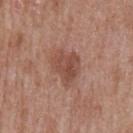Recorded during total-body skin imaging; not selected for excision or biopsy.
On the mid back.
Automated image analysis of the tile measured an eccentricity of roughly 0.65 and two-axis asymmetry of about 0.35.
Cropped from a whole-body photographic skin survey; the tile spans about 15 mm.
Approximately 4 mm at its widest.
A male patient, in their mid-60s.